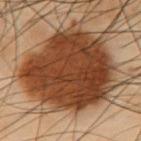<tbp_lesion>
<biopsy_status>not biopsied; imaged during a skin examination</biopsy_status>
<image>
  <source>total-body photography crop</source>
  <field_of_view_mm>15</field_of_view_mm>
</image>
<patient>
  <sex>male</sex>
  <age_approx>55</age_approx>
</patient>
<lighting>cross-polarized</lighting>
<lesion_size>
  <long_diameter_mm_approx>10.0</long_diameter_mm_approx>
</lesion_size>
<site>chest</site>
</tbp_lesion>